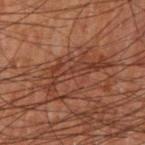Q: Is there a histopathology result?
A: catalogued during a skin exam; not biopsied
Q: Who is the patient?
A: male, aged around 70
Q: What did automated image analysis measure?
A: a lesion color around L≈29 a*≈19 b*≈23 in CIELAB, roughly 5 lightness units darker than nearby skin, and a normalized border contrast of about 6; an automated nevus-likeness rating near 0 out of 100 and a lesion-detection confidence of about 75/100
Q: Where on the body is the lesion?
A: the right forearm
Q: What is the imaging modality?
A: ~15 mm tile from a whole-body skin photo
Q: Lesion size?
A: ≈7.5 mm
Q: Illumination type?
A: cross-polarized